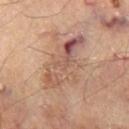Impression: Recorded during total-body skin imaging; not selected for excision or biopsy. Acquisition and patient details: A roughly 15 mm field-of-view crop from a total-body skin photograph. The patient is a male roughly 70 years of age. Located on the leg.